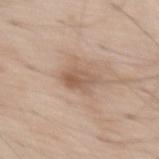<record>
  <automated_metrics>
    <area_mm2_approx>6.0</area_mm2_approx>
    <eccentricity>0.75</eccentricity>
    <cielab_L>57</cielab_L>
    <cielab_a>17</cielab_a>
    <cielab_b>29</cielab_b>
    <vs_skin_darker_L>9.0</vs_skin_darker_L>
    <nevus_likeness_0_100>0</nevus_likeness_0_100>
    <lesion_detection_confidence_0_100>100</lesion_detection_confidence_0_100>
  </automated_metrics>
  <lesion_size>
    <long_diameter_mm_approx>3.5</long_diameter_mm_approx>
  </lesion_size>
  <image>
    <source>total-body photography crop</source>
    <field_of_view_mm>15</field_of_view_mm>
  </image>
  <site>abdomen</site>
  <patient>
    <sex>male</sex>
    <age_approx>70</age_approx>
  </patient>
  <lighting>white-light</lighting>
</record>